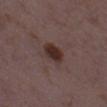This lesion was catalogued during total-body skin photography and was not selected for biopsy. Automated image analysis of the tile measured an outline eccentricity of about 0.7 (0 = round, 1 = elongated) and a symmetry-axis asymmetry near 0.2. And it measured an average lesion color of about L≈29 a*≈17 b*≈19 (CIELAB), a lesion–skin lightness drop of about 11, and a normalized lesion–skin contrast near 10.5. And it measured a classifier nevus-likeness of about 95/100 and a detector confidence of about 100 out of 100 that the crop contains a lesion. From the left thigh. The subject is a female aged around 35. Cropped from a whole-body photographic skin survey; the tile spans about 15 mm. The lesion's longest dimension is about 3 mm.